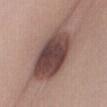| field | value |
|---|---|
| biopsy status | catalogued during a skin exam; not biopsied |